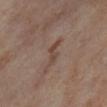No biopsy was performed on this lesion — it was imaged during a full skin examination and was not determined to be concerning. A close-up tile cropped from a whole-body skin photograph, about 15 mm across. A female subject, aged 53 to 57. The lesion is located on the right thigh. Measured at roughly 3 mm in maximum diameter.